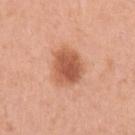<record>
  <biopsy_status>not biopsied; imaged during a skin examination</biopsy_status>
  <site>left upper arm</site>
  <image>
    <source>total-body photography crop</source>
    <field_of_view_mm>15</field_of_view_mm>
  </image>
  <lighting>white-light</lighting>
  <lesion_size>
    <long_diameter_mm_approx>4.0</long_diameter_mm_approx>
  </lesion_size>
  <patient>
    <sex>female</sex>
    <age_approx>40</age_approx>
  </patient>
</record>